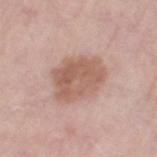This lesion was catalogued during total-body skin photography and was not selected for biopsy. An algorithmic analysis of the crop reported a footprint of about 20 mm², a shape eccentricity near 0.6, and two-axis asymmetry of about 0.15. The analysis additionally found a mean CIELAB color near L≈59 a*≈20 b*≈27, roughly 10 lightness units darker than nearby skin, and a lesion-to-skin contrast of about 7 (normalized; higher = more distinct). The analysis additionally found a border-irregularity rating of about 1.5/10 and peripheral color asymmetry of about 1.5. On the left thigh. The patient is a female aged 68–72. This image is a 15 mm lesion crop taken from a total-body photograph.